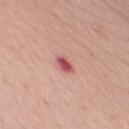The lesion was tiled from a total-body skin photograph and was not biopsied.
An algorithmic analysis of the crop reported a lesion area of about 3 mm², an outline eccentricity of about 0.9 (0 = round, 1 = elongated), and a symmetry-axis asymmetry near 0.2. The analysis additionally found an average lesion color of about L≈54 a*≈33 b*≈23 (CIELAB), about 15 CIELAB-L* units darker than the surrounding skin, and a normalized border contrast of about 9.5. The analysis additionally found a classifier nevus-likeness of about 0/100.
The recorded lesion diameter is about 3 mm.
Captured under white-light illumination.
The subject is a male in their 60s.
From the chest.
A lesion tile, about 15 mm wide, cut from a 3D total-body photograph.